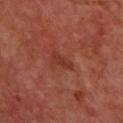Findings:
* workup: catalogued during a skin exam; not biopsied
* TBP lesion metrics: an area of roughly 3 mm², an outline eccentricity of about 0.85 (0 = round, 1 = elongated), and a symmetry-axis asymmetry near 0.4
* image source: ~15 mm crop, total-body skin-cancer survey
* site: the upper back
* diameter: about 3 mm
* subject: male, approximately 65 years of age